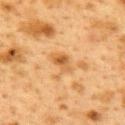| field | value |
|---|---|
| workup | imaged on a skin check; not biopsied |
| location | the upper back |
| subject | female, aged around 40 |
| illumination | cross-polarized illumination |
| lesion diameter | ≈3 mm |
| acquisition | total-body-photography crop, ~15 mm field of view |